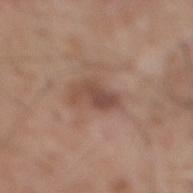| feature | finding |
|---|---|
| workup | no biopsy performed (imaged during a skin exam) |
| subject | male, about 60 years old |
| site | the mid back |
| imaging modality | ~15 mm crop, total-body skin-cancer survey |